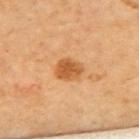This lesion was catalogued during total-body skin photography and was not selected for biopsy.
Imaged with cross-polarized lighting.
From the upper back.
A patient in their 70s.
Cropped from a whole-body photographic skin survey; the tile spans about 15 mm.